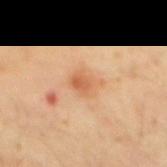Case summary:
* notes · catalogued during a skin exam; not biopsied
* body site · the back
* imaging modality · 15 mm crop, total-body photography
* subject · male, aged 28 to 32